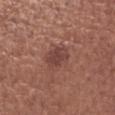Captured during whole-body skin photography for melanoma surveillance; the lesion was not biopsied. The tile uses white-light illumination. Approximately 3.5 mm at its widest. Located on the arm. A female subject, aged 63 to 67. A 15 mm close-up tile from a total-body photography series done for melanoma screening.